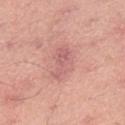The lesion was tiled from a total-body skin photograph and was not biopsied. The subject is a male in their 50s. Approximately 4.5 mm at its widest. From the right thigh. Captured under white-light illumination. The total-body-photography lesion software estimated a lesion area of about 9.5 mm² and a shape-asymmetry score of about 0.3 (0 = symmetric). And it measured a nevus-likeness score of about 0/100 and lesion-presence confidence of about 100/100. A 15 mm close-up tile from a total-body photography series done for melanoma screening.